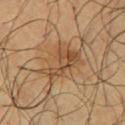Assessment:
This lesion was catalogued during total-body skin photography and was not selected for biopsy.
Acquisition and patient details:
Captured under cross-polarized illumination. The recorded lesion diameter is about 6 mm. This image is a 15 mm lesion crop taken from a total-body photograph. The patient is a male aged 63–67. An algorithmic analysis of the crop reported an area of roughly 13 mm² and an outline eccentricity of about 0.85 (0 = round, 1 = elongated). The lesion is located on the left thigh.